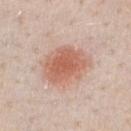<record>
<biopsy_status>not biopsied; imaged during a skin examination</biopsy_status>
<image>
  <source>total-body photography crop</source>
  <field_of_view_mm>15</field_of_view_mm>
</image>
<patient>
  <sex>male</sex>
  <age_approx>40</age_approx>
</patient>
<site>chest</site>
</record>